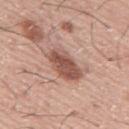Findings:
• biopsy status · total-body-photography surveillance lesion; no biopsy
• patient · male, aged 73 to 77
• image source · 15 mm crop, total-body photography
• site · the mid back